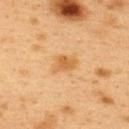This lesion was catalogued during total-body skin photography and was not selected for biopsy. On the upper back. Approximately 3 mm at its widest. This is a cross-polarized tile. A female subject, approximately 40 years of age. A roughly 15 mm field-of-view crop from a total-body skin photograph.